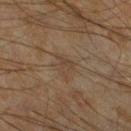Q: Was this lesion biopsied?
A: catalogued during a skin exam; not biopsied
Q: How was the tile lit?
A: cross-polarized
Q: How was this image acquired?
A: 15 mm crop, total-body photography
Q: Lesion location?
A: the left lower leg
Q: What are the patient's age and sex?
A: male, aged approximately 45
Q: Lesion size?
A: ≈3.5 mm